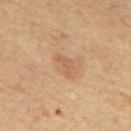The lesion was tiled from a total-body skin photograph and was not biopsied. This is a cross-polarized tile. Cropped from a whole-body photographic skin survey; the tile spans about 15 mm. On the mid back. The patient is a male aged 63 to 67. The total-body-photography lesion software estimated radial color variation of about 0. The analysis additionally found a detector confidence of about 100 out of 100 that the crop contains a lesion. The lesion's longest dimension is about 3 mm.